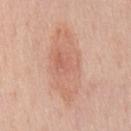Q: Is there a histopathology result?
A: imaged on a skin check; not biopsied
Q: What is the lesion's diameter?
A: ≈9 mm
Q: What are the patient's age and sex?
A: male, aged 48 to 52
Q: How was the tile lit?
A: white-light illumination
Q: What is the imaging modality?
A: ~15 mm tile from a whole-body skin photo
Q: What is the anatomic site?
A: the chest